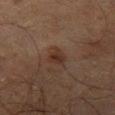A roughly 15 mm field-of-view crop from a total-body skin photograph. A male subject, aged 63–67. From the left thigh. The tile uses cross-polarized illumination.